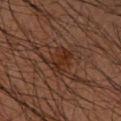Part of a total-body skin-imaging series; this lesion was reviewed on a skin check and was not flagged for biopsy.
Captured under cross-polarized illumination.
From the left forearm.
Automated tile analysis of the lesion measured an area of roughly 4.5 mm², an outline eccentricity of about 0.9 (0 = round, 1 = elongated), and two-axis asymmetry of about 0.4. The software also gave a lesion color around L≈25 a*≈19 b*≈25 in CIELAB and a normalized border contrast of about 7. It also reported a nevus-likeness score of about 5/100 and a lesion-detection confidence of about 90/100.
The patient is a male aged around 60.
Cropped from a whole-body photographic skin survey; the tile spans about 15 mm.